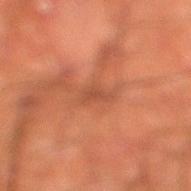biopsy status — total-body-photography surveillance lesion; no biopsy | diameter — about 3 mm | patient — male, aged approximately 50 | imaging modality — ~15 mm tile from a whole-body skin photo | location — the leg | automated lesion analysis — an area of roughly 3 mm² and a shape eccentricity near 0.9; a lesion color around L≈40 a*≈23 b*≈29 in CIELAB, a lesion–skin lightness drop of about 6, and a normalized border contrast of about 5.5; a border-irregularity rating of about 4/10, internal color variation of about 0.5 on a 0–10 scale, and a peripheral color-asymmetry measure near 0.5.